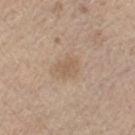Captured under white-light illumination.
The lesion is located on the left lower leg.
Automated tile analysis of the lesion measured a lesion area of about 4.5 mm² and a shape-asymmetry score of about 0.25 (0 = symmetric). The software also gave a nevus-likeness score of about 5/100.
A male patient approximately 70 years of age.
This image is a 15 mm lesion crop taken from a total-body photograph.